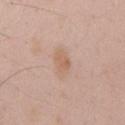workup: imaged on a skin check; not biopsied | acquisition: ~15 mm crop, total-body skin-cancer survey | diameter: about 3 mm | location: the chest | TBP lesion metrics: a lesion–skin lightness drop of about 7 and a normalized lesion–skin contrast near 5.5; lesion-presence confidence of about 100/100 | lighting: white-light | subject: male, aged 48–52.